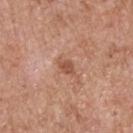Case summary:
* diameter: ≈2.5 mm
* subject: male, roughly 60 years of age
* image source: ~15 mm crop, total-body skin-cancer survey
* anatomic site: the back
* tile lighting: white-light illumination